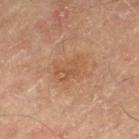Clinical impression: This lesion was catalogued during total-body skin photography and was not selected for biopsy. Clinical summary: A lesion tile, about 15 mm wide, cut from a 3D total-body photograph. The lesion-visualizer software estimated a nevus-likeness score of about 0/100 and a lesion-detection confidence of about 100/100. The lesion is located on the leg. A male patient about 70 years old. About 4 mm across.